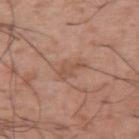Impression: No biopsy was performed on this lesion — it was imaged during a full skin examination and was not determined to be concerning. Clinical summary: A male patient, aged approximately 55. The lesion's longest dimension is about 3 mm. Imaged with white-light lighting. On the arm. The lesion-visualizer software estimated a footprint of about 3.5 mm², an outline eccentricity of about 0.85 (0 = round, 1 = elongated), and a shape-asymmetry score of about 0.55 (0 = symmetric). This image is a 15 mm lesion crop taken from a total-body photograph.